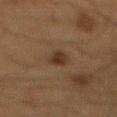workup: imaged on a skin check; not biopsied | location: the mid back | subject: male, aged 63 to 67 | image: total-body-photography crop, ~15 mm field of view.